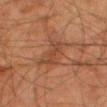notes: imaged on a skin check; not biopsied
acquisition: ~15 mm crop, total-body skin-cancer survey
tile lighting: cross-polarized
size: about 4 mm
body site: the right thigh
automated lesion analysis: an outline eccentricity of about 0.9 (0 = round, 1 = elongated) and a shape-asymmetry score of about 0.45 (0 = symmetric); an average lesion color of about L≈34 a*≈19 b*≈26 (CIELAB) and a normalized lesion–skin contrast near 5.5
patient: male, about 80 years old